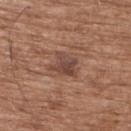• biopsy status: catalogued during a skin exam; not biopsied
• lesion size: ~3.5 mm (longest diameter)
• patient: male, aged approximately 75
• location: the upper back
• illumination: white-light illumination
• image-analysis metrics: an area of roughly 6.5 mm², an eccentricity of roughly 0.5, and a shape-asymmetry score of about 0.4 (0 = symmetric); a mean CIELAB color near L≈45 a*≈20 b*≈25 and about 9 CIELAB-L* units darker than the surrounding skin; a border-irregularity rating of about 4.5/10, internal color variation of about 4.5 on a 0–10 scale, and a peripheral color-asymmetry measure near 1.5
• image source: 15 mm crop, total-body photography